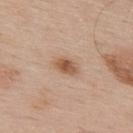<case>
<biopsy_status>not biopsied; imaged during a skin examination</biopsy_status>
<lesion_size>
  <long_diameter_mm_approx>3.0</long_diameter_mm_approx>
</lesion_size>
<site>upper back</site>
<lighting>white-light</lighting>
<image>
  <source>total-body photography crop</source>
  <field_of_view_mm>15</field_of_view_mm>
</image>
<patient>
  <sex>male</sex>
  <age_approx>55</age_approx>
</patient>
<automated_metrics>
  <cielab_L>55</cielab_L>
  <cielab_a>20</cielab_a>
  <cielab_b>32</cielab_b>
  <vs_skin_darker_L>12.0</vs_skin_darker_L>
  <border_irregularity_0_10>2.5</border_irregularity_0_10>
  <color_variation_0_10>3.5</color_variation_0_10>
  <peripheral_color_asymmetry>1.0</peripheral_color_asymmetry>
</automated_metrics>
</case>